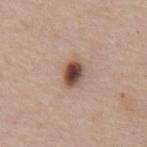workup — total-body-photography surveillance lesion; no biopsy | patient — male, roughly 55 years of age | automated lesion analysis — a footprint of about 5 mm², a shape eccentricity near 0.7, and a symmetry-axis asymmetry near 0.2; roughly 18 lightness units darker than nearby skin and a lesion-to-skin contrast of about 12.5 (normalized; higher = more distinct); a color-variation rating of about 7.5/10 and radial color variation of about 2; an automated nevus-likeness rating near 100 out of 100 | body site — the abdomen | image source — 15 mm crop, total-body photography.